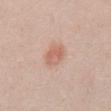Impression:
Part of a total-body skin-imaging series; this lesion was reviewed on a skin check and was not flagged for biopsy.
Clinical summary:
The subject is a male about 50 years old. This is a white-light tile. Cropped from a total-body skin-imaging series; the visible field is about 15 mm. Approximately 3 mm at its widest. The lesion is located on the abdomen.